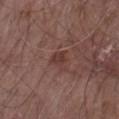Assessment:
Imaged during a routine full-body skin examination; the lesion was not biopsied and no histopathology is available.
Acquisition and patient details:
Measured at roughly 3 mm in maximum diameter. A region of skin cropped from a whole-body photographic capture, roughly 15 mm wide. This is a white-light tile. The subject is a male approximately 65 years of age. From the arm. Automated tile analysis of the lesion measured an eccentricity of roughly 0.65. The analysis additionally found a border-irregularity rating of about 3/10, a within-lesion color-variation index near 1.5/10, and radial color variation of about 0.5. The analysis additionally found a nevus-likeness score of about 0/100 and a detector confidence of about 100 out of 100 that the crop contains a lesion.